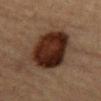The lesion's longest dimension is about 6.5 mm.
Cropped from a total-body skin-imaging series; the visible field is about 15 mm.
On the abdomen.
The patient is a female aged approximately 60.